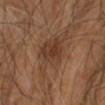Context: A 15 mm close-up tile from a total-body photography series done for melanoma screening. The patient is a male approximately 60 years of age. The recorded lesion diameter is about 7 mm. This is a cross-polarized tile. The lesion is located on the right arm.